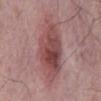Clinical impression:
The lesion was photographed on a routine skin check and not biopsied; there is no pathology result.
Context:
The lesion is located on the mid back. A region of skin cropped from a whole-body photographic capture, roughly 15 mm wide. The patient is a male aged around 65.